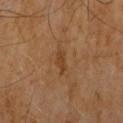<lesion>
  <biopsy_status>not biopsied; imaged during a skin examination</biopsy_status>
  <lighting>cross-polarized</lighting>
  <site>front of the torso</site>
  <lesion_size>
    <long_diameter_mm_approx>2.5</long_diameter_mm_approx>
  </lesion_size>
  <image>
    <source>total-body photography crop</source>
    <field_of_view_mm>15</field_of_view_mm>
  </image>
  <patient>
    <sex>male</sex>
    <age_approx>60</age_approx>
  </patient>
</lesion>